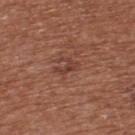The lesion was tiled from a total-body skin photograph and was not biopsied.
A male subject, aged approximately 65.
From the upper back.
This image is a 15 mm lesion crop taken from a total-body photograph.
Longest diameter approximately 3 mm.
The total-body-photography lesion software estimated a mean CIELAB color near L≈40 a*≈24 b*≈27, roughly 8 lightness units darker than nearby skin, and a normalized lesion–skin contrast near 6.5. The software also gave border irregularity of about 6 on a 0–10 scale, a color-variation rating of about 0/10, and radial color variation of about 0. The software also gave a nevus-likeness score of about 0/100.